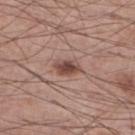biopsy status: catalogued during a skin exam; not biopsied
diameter: ~3.5 mm (longest diameter)
image: ~15 mm tile from a whole-body skin photo
patient: male, aged around 60
automated metrics: an area of roughly 5.5 mm² and a shape-asymmetry score of about 0.2 (0 = symmetric); an average lesion color of about L≈47 a*≈19 b*≈24 (CIELAB) and a lesion-to-skin contrast of about 9 (normalized; higher = more distinct); a border-irregularity rating of about 2/10, internal color variation of about 4 on a 0–10 scale, and radial color variation of about 1; an automated nevus-likeness rating near 90 out of 100 and a detector confidence of about 100 out of 100 that the crop contains a lesion
body site: the right lower leg
tile lighting: white-light